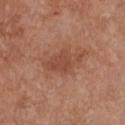workup=catalogued during a skin exam; not biopsied | imaging modality=15 mm crop, total-body photography | patient=female, aged 73–77 | body site=the chest.